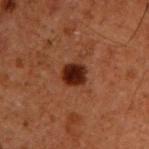The lesion was photographed on a routine skin check and not biopsied; there is no pathology result.
This is a cross-polarized tile.
On the back.
A male patient aged 58 to 62.
A lesion tile, about 15 mm wide, cut from a 3D total-body photograph.